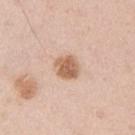Recorded during total-body skin imaging; not selected for excision or biopsy. From the arm. A 15 mm crop from a total-body photograph taken for skin-cancer surveillance. The tile uses white-light illumination. Longest diameter approximately 3 mm. Automated tile analysis of the lesion measured a footprint of about 7 mm², a shape eccentricity near 0.4, and a symmetry-axis asymmetry near 0.2. And it measured a mean CIELAB color near L≈62 a*≈20 b*≈32 and a lesion–skin lightness drop of about 13. A male patient aged around 40.